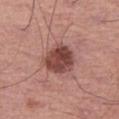<record>
<patient>
  <sex>male</sex>
  <age_approx>65</age_approx>
</patient>
<image>
  <source>total-body photography crop</source>
  <field_of_view_mm>15</field_of_view_mm>
</image>
<site>right thigh</site>
</record>